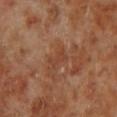| feature | finding |
|---|---|
| biopsy status | total-body-photography surveillance lesion; no biopsy |
| size | ~2.5 mm (longest diameter) |
| site | the left lower leg |
| image | total-body-photography crop, ~15 mm field of view |
| patient | male, approximately 70 years of age |